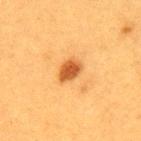The lesion was photographed on a routine skin check and not biopsied; there is no pathology result.
Captured under cross-polarized illumination.
On the upper back.
A female subject aged 38 to 42.
Cropped from a whole-body photographic skin survey; the tile spans about 15 mm.